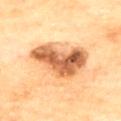{"biopsy_status": "not biopsied; imaged during a skin examination", "lesion_size": {"long_diameter_mm_approx": 6.5}, "site": "upper back", "lighting": "cross-polarized", "automated_metrics": {"border_irregularity_0_10": 4.0, "color_variation_0_10": 9.0}, "patient": {"sex": "female", "age_approx": 80}, "image": {"source": "total-body photography crop", "field_of_view_mm": 15}}